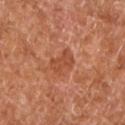Q: Was a biopsy performed?
A: total-body-photography surveillance lesion; no biopsy
Q: What is the lesion's diameter?
A: ≈3.5 mm
Q: Illumination type?
A: cross-polarized illumination
Q: What is the anatomic site?
A: the left lower leg
Q: What are the patient's age and sex?
A: aged around 65
Q: What is the imaging modality?
A: 15 mm crop, total-body photography